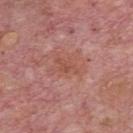The lesion was photographed on a routine skin check and not biopsied; there is no pathology result. A male patient, aged 73 to 77. About 3 mm across. Automated image analysis of the tile measured a symmetry-axis asymmetry near 0.45. The software also gave a classifier nevus-likeness of about 0/100 and lesion-presence confidence of about 100/100. This image is a 15 mm lesion crop taken from a total-body photograph. The lesion is on the chest. Captured under white-light illumination.